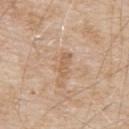workup = no biopsy performed (imaged during a skin exam) | illumination = white-light illumination | image source = 15 mm crop, total-body photography | patient = male, aged 78 to 82 | image-analysis metrics = a lesion area of about 3 mm², an eccentricity of roughly 0.9, and two-axis asymmetry of about 0.4; a mean CIELAB color near L≈60 a*≈18 b*≈33, a lesion–skin lightness drop of about 8, and a normalized border contrast of about 5.5; border irregularity of about 4.5 on a 0–10 scale, a color-variation rating of about 0/10, and radial color variation of about 0; a classifier nevus-likeness of about 0/100 | lesion diameter = about 3 mm | anatomic site = the upper back.